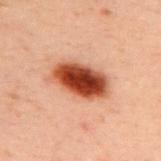The lesion was photographed on a routine skin check and not biopsied; there is no pathology result. Measured at roughly 6 mm in maximum diameter. A male subject in their 40s. The lesion is on the upper back. A close-up tile cropped from a whole-body skin photograph, about 15 mm across. The total-body-photography lesion software estimated a lesion color around L≈39 a*≈26 b*≈30 in CIELAB, roughly 18 lightness units darker than nearby skin, and a normalized border contrast of about 14. The software also gave a border-irregularity index near 2/10, internal color variation of about 7.5 on a 0–10 scale, and radial color variation of about 2. The tile uses cross-polarized illumination.